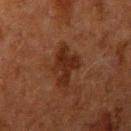Assessment: No biopsy was performed on this lesion — it was imaged during a full skin examination and was not determined to be concerning.